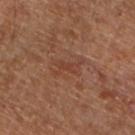<lesion>
<biopsy_status>not biopsied; imaged during a skin examination</biopsy_status>
<lesion_size>
  <long_diameter_mm_approx>4.0</long_diameter_mm_approx>
</lesion_size>
<image>
  <source>total-body photography crop</source>
  <field_of_view_mm>15</field_of_view_mm>
</image>
<site>left lower leg</site>
<automated_metrics>
  <border_irregularity_0_10>7.0</border_irregularity_0_10>
  <color_variation_0_10>1.0</color_variation_0_10>
  <peripheral_color_asymmetry>0.0</peripheral_color_asymmetry>
  <nevus_likeness_0_100>0</nevus_likeness_0_100>
</automated_metrics>
<patient>
  <sex>male</sex>
  <age_approx>60</age_approx>
</patient>
</lesion>